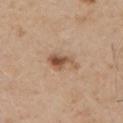{
  "automated_metrics": {
    "area_mm2_approx": 6.0,
    "eccentricity": 0.85,
    "shape_asymmetry": 0.35,
    "vs_skin_darker_L": 12.0,
    "border_irregularity_0_10": 4.5,
    "peripheral_color_asymmetry": 1.5
  },
  "patient": {
    "sex": "male",
    "age_approx": 50
  },
  "lesion_size": {
    "long_diameter_mm_approx": 4.0
  },
  "image": {
    "source": "total-body photography crop",
    "field_of_view_mm": 15
  },
  "lighting": "white-light",
  "site": "chest"
}